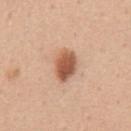Assessment:
The lesion was photographed on a routine skin check and not biopsied; there is no pathology result.
Background:
The lesion is located on the chest. A male subject, aged around 50. Imaged with white-light lighting. A roughly 15 mm field-of-view crop from a total-body skin photograph.